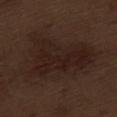automated_metrics:
  area_mm2_approx: 40.0
  eccentricity: 0.75
  shape_asymmetry: 0.4
  cielab_L: 20
  cielab_a: 15
  cielab_b: 19
  vs_skin_darker_L: 5.0
  vs_skin_contrast_norm: 7.5
patient:
  sex: male
  age_approx: 70
site: left thigh
image:
  source: total-body photography crop
  field_of_view_mm: 15
lesion_size:
  long_diameter_mm_approx: 9.0
lighting: white-light